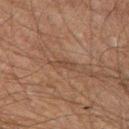Imaged during a routine full-body skin examination; the lesion was not biopsied and no histopathology is available.
A male subject approximately 60 years of age.
The lesion's longest dimension is about 2.5 mm.
An algorithmic analysis of the crop reported a detector confidence of about 70 out of 100 that the crop contains a lesion.
Cropped from a whole-body photographic skin survey; the tile spans about 15 mm.
Located on the leg.
This is a cross-polarized tile.